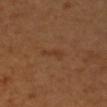Case summary:
• biopsy status · total-body-photography surveillance lesion; no biopsy
• image-analysis metrics · a lesion area of about 2.5 mm² and two-axis asymmetry of about 0.5; roughly 5 lightness units darker than nearby skin and a normalized border contrast of about 5
• patient · female, in their mid- to late 50s
• tile lighting · cross-polarized
• location · the right forearm
• image · 15 mm crop, total-body photography
• diameter · about 2.5 mm